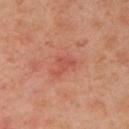This lesion was catalogued during total-body skin photography and was not selected for biopsy. The tile uses cross-polarized illumination. The patient is a female aged approximately 40. Located on the left arm. Cropped from a total-body skin-imaging series; the visible field is about 15 mm. The total-body-photography lesion software estimated a lesion area of about 3.5 mm² and two-axis asymmetry of about 0.55. The recorded lesion diameter is about 3 mm.